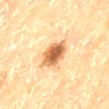Q: Was this lesion biopsied?
A: imaged on a skin check; not biopsied
Q: Lesion size?
A: ≈4 mm
Q: Patient demographics?
A: male, aged 83 or older
Q: Lesion location?
A: the back
Q: Illumination type?
A: cross-polarized illumination
Q: How was this image acquired?
A: 15 mm crop, total-body photography